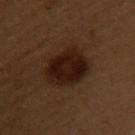| key | value |
|---|---|
| follow-up | no biopsy performed (imaged during a skin exam) |
| size | ~6 mm (longest diameter) |
| site | the upper back |
| automated metrics | a footprint of about 18 mm², a shape eccentricity near 0.7, and two-axis asymmetry of about 0.15; a lesion color around L≈15 a*≈15 b*≈18 in CIELAB, a lesion–skin lightness drop of about 8, and a normalized lesion–skin contrast near 11.5 |
| tile lighting | cross-polarized illumination |
| patient | female, aged 48 to 52 |
| image source | ~15 mm tile from a whole-body skin photo |